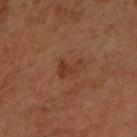Captured during whole-body skin photography for melanoma surveillance; the lesion was not biopsied.
The subject is female.
A roughly 15 mm field-of-view crop from a total-body skin photograph.
From the left forearm.